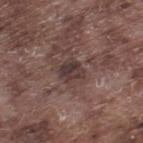Q: Was a biopsy performed?
A: no biopsy performed (imaged during a skin exam)
Q: How was this image acquired?
A: total-body-photography crop, ~15 mm field of view
Q: Where on the body is the lesion?
A: the leg
Q: How was the tile lit?
A: white-light
Q: Patient demographics?
A: male, approximately 75 years of age